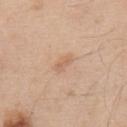Imaged during a routine full-body skin examination; the lesion was not biopsied and no histopathology is available. A male subject, in their mid- to late 50s. From the upper back. A 15 mm crop from a total-body photograph taken for skin-cancer surveillance.